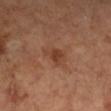The lesion is located on the left lower leg.
About 2.5 mm across.
A male patient, roughly 65 years of age.
Imaged with cross-polarized lighting.
This image is a 15 mm lesion crop taken from a total-body photograph.